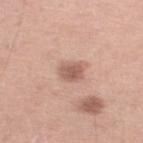The lesion was photographed on a routine skin check and not biopsied; there is no pathology result.